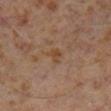Clinical impression:
Part of a total-body skin-imaging series; this lesion was reviewed on a skin check and was not flagged for biopsy.
Acquisition and patient details:
Automated tile analysis of the lesion measured a footprint of about 3.5 mm², an outline eccentricity of about 0.7 (0 = round, 1 = elongated), and two-axis asymmetry of about 0.4. The analysis additionally found an automated nevus-likeness rating near 0 out of 100. A close-up tile cropped from a whole-body skin photograph, about 15 mm across. A male subject roughly 60 years of age. This is a cross-polarized tile. The lesion's longest dimension is about 2.5 mm. From the right lower leg.